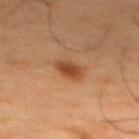No biopsy was performed on this lesion — it was imaged during a full skin examination and was not determined to be concerning.
From the upper back.
The lesion's longest dimension is about 3 mm.
A male subject, aged 58 to 62.
A region of skin cropped from a whole-body photographic capture, roughly 15 mm wide.
The tile uses cross-polarized illumination.